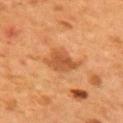Image and clinical context: This image is a 15 mm lesion crop taken from a total-body photograph. A male patient, approximately 55 years of age. From the mid back.